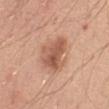  biopsy_status: not biopsied; imaged during a skin examination
  patient:
    sex: male
    age_approx: 50
  site: abdomen
  lesion_size:
    long_diameter_mm_approx: 4.5
  image:
    source: total-body photography crop
    field_of_view_mm: 15